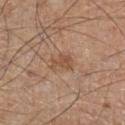biopsy status: total-body-photography surveillance lesion; no biopsy | tile lighting: white-light illumination | image source: 15 mm crop, total-body photography | subject: male, in their mid- to late 60s | automated lesion analysis: an area of roughly 4.5 mm² and an eccentricity of roughly 0.75; a border-irregularity rating of about 3.5/10, a within-lesion color-variation index near 1.5/10, and radial color variation of about 0.5; an automated nevus-likeness rating near 10 out of 100 and a lesion-detection confidence of about 100/100 | site: the left lower leg | diameter: ≈2.5 mm.